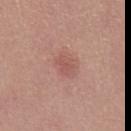<record>
  <biopsy_status>not biopsied; imaged during a skin examination</biopsy_status>
  <lesion_size>
    <long_diameter_mm_approx>2.5</long_diameter_mm_approx>
  </lesion_size>
  <image>
    <source>total-body photography crop</source>
    <field_of_view_mm>15</field_of_view_mm>
  </image>
  <lighting>white-light</lighting>
  <site>chest</site>
  <patient>
    <sex>female</sex>
    <age_approx>35</age_approx>
  </patient>
</record>